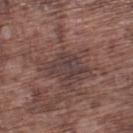{"biopsy_status": "not biopsied; imaged during a skin examination", "patient": {"sex": "male", "age_approx": 75}, "lesion_size": {"long_diameter_mm_approx": 4.5}, "lighting": "white-light", "automated_metrics": {"eccentricity": 0.7, "shape_asymmetry": 0.4, "vs_skin_darker_L": 7.0, "nevus_likeness_0_100": 5, "lesion_detection_confidence_0_100": 75}, "site": "left lower leg", "image": {"source": "total-body photography crop", "field_of_view_mm": 15}}